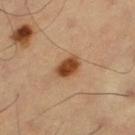biopsy status: total-body-photography surveillance lesion; no biopsy
illumination: cross-polarized illumination
lesion diameter: ~3 mm (longest diameter)
subject: male, in their 60s
image source: ~15 mm tile from a whole-body skin photo
location: the left thigh
image-analysis metrics: roughly 15 lightness units darker than nearby skin and a lesion-to-skin contrast of about 11 (normalized; higher = more distinct); a border-irregularity rating of about 1.5/10; lesion-presence confidence of about 100/100